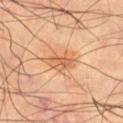This lesion was catalogued during total-body skin photography and was not selected for biopsy.
Located on the right thigh.
Cropped from a whole-body photographic skin survey; the tile spans about 15 mm.
An algorithmic analysis of the crop reported a border-irregularity rating of about 3.5/10, a within-lesion color-variation index near 3.5/10, and peripheral color asymmetry of about 1.5.
The subject is a male approximately 65 years of age.
The lesion's longest dimension is about 4.5 mm.
Captured under cross-polarized illumination.